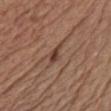Assessment:
The lesion was photographed on a routine skin check and not biopsied; there is no pathology result.
Acquisition and patient details:
The recorded lesion diameter is about 3 mm. The lesion is located on the chest. The subject is a male approximately 55 years of age. A lesion tile, about 15 mm wide, cut from a 3D total-body photograph.